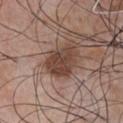{"site": "chest", "patient": {"sex": "male", "age_approx": 70}, "image": {"source": "total-body photography crop", "field_of_view_mm": 15}}